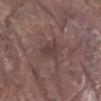The lesion was tiled from a total-body skin photograph and was not biopsied.
A 15 mm close-up tile from a total-body photography series done for melanoma screening.
A male subject, about 80 years old.
An algorithmic analysis of the crop reported a lesion area of about 4.5 mm², an outline eccentricity of about 0.75 (0 = round, 1 = elongated), and a shape-asymmetry score of about 0.3 (0 = symmetric). The analysis additionally found a nevus-likeness score of about 0/100 and a lesion-detection confidence of about 100/100.
The lesion is located on the right upper arm.
The tile uses white-light illumination.